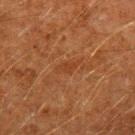* follow-up · catalogued during a skin exam; not biopsied
* image · total-body-photography crop, ~15 mm field of view
* illumination · cross-polarized illumination
* subject · male, approximately 60 years of age
* automated lesion analysis · a nevus-likeness score of about 0/100 and lesion-presence confidence of about 90/100
* diameter · ≈3 mm
* anatomic site · the left upper arm